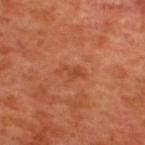workup: imaged on a skin check; not biopsied | patient: male, aged around 50 | image source: ~15 mm tile from a whole-body skin photo | site: the upper back.